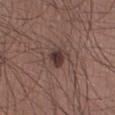Findings:
- workup — catalogued during a skin exam; not biopsied
- lighting — white-light illumination
- TBP lesion metrics — a border-irregularity rating of about 2.5/10, a within-lesion color-variation index near 3/10, and a peripheral color-asymmetry measure near 1; a nevus-likeness score of about 90/100 and lesion-presence confidence of about 100/100
- location — the right lower leg
- patient — male, approximately 35 years of age
- image — total-body-photography crop, ~15 mm field of view